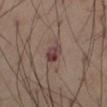Clinical impression:
This lesion was catalogued during total-body skin photography and was not selected for biopsy.
Context:
The lesion-visualizer software estimated a lesion area of about 3.5 mm², an eccentricity of roughly 0.8, and a symmetry-axis asymmetry near 0.25. The software also gave a nevus-likeness score of about 0/100. A roughly 15 mm field-of-view crop from a total-body skin photograph. A male patient, aged around 55. On the right thigh. The lesion's longest dimension is about 3 mm. Captured under cross-polarized illumination.